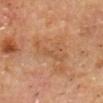follow-up: no biopsy performed (imaged during a skin exam) | subject: male, aged around 60 | tile lighting: cross-polarized illumination | size: about 5.5 mm | body site: the head or neck | TBP lesion metrics: a footprint of about 8.5 mm² and a shape-asymmetry score of about 0.5 (0 = symmetric); a border-irregularity rating of about 7.5/10, a within-lesion color-variation index near 2.5/10, and radial color variation of about 1; a nevus-likeness score of about 0/100 and a lesion-detection confidence of about 100/100 | acquisition: ~15 mm tile from a whole-body skin photo.